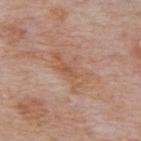{
  "biopsy_status": "not biopsied; imaged during a skin examination",
  "lighting": "white-light",
  "lesion_size": {
    "long_diameter_mm_approx": 5.0
  },
  "image": {
    "source": "total-body photography crop",
    "field_of_view_mm": 15
  },
  "patient": {
    "sex": "male",
    "age_approx": 75
  },
  "site": "back"
}